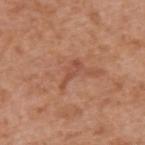Notes:
- patient: male, in their mid- to late 60s
- image: ~15 mm crop, total-body skin-cancer survey
- site: the back